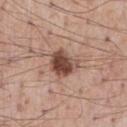Part of a total-body skin-imaging series; this lesion was reviewed on a skin check and was not flagged for biopsy. Cropped from a total-body skin-imaging series; the visible field is about 15 mm. Longest diameter approximately 3.5 mm. Imaged with white-light lighting. A male patient aged 53 to 57. The lesion is on the chest.